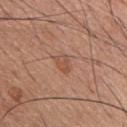| field | value |
|---|---|
| biopsy status | no biopsy performed (imaged during a skin exam) |
| subject | male, aged 58–62 |
| site | the chest |
| image | ~15 mm tile from a whole-body skin photo |